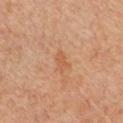workup=no biopsy performed (imaged during a skin exam)
image=~15 mm crop, total-body skin-cancer survey
subject=female, aged 28 to 32
body site=the chest